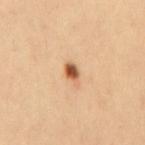Part of a total-body skin-imaging series; this lesion was reviewed on a skin check and was not flagged for biopsy. On the mid back. About 2.5 mm across. The tile uses cross-polarized illumination. Cropped from a total-body skin-imaging series; the visible field is about 15 mm. A male patient, roughly 35 years of age. Automated image analysis of the tile measured a lesion area of about 3.5 mm², a shape eccentricity near 0.8, and two-axis asymmetry of about 0.3. And it measured lesion-presence confidence of about 100/100.